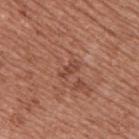image source = total-body-photography crop, ~15 mm field of view; illumination = white-light illumination; lesion size = ≈2.5 mm; subject = male, aged 53–57; site = the upper back.